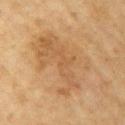This lesion was catalogued during total-body skin photography and was not selected for biopsy. The lesion is located on the right upper arm. This is a cross-polarized tile. The lesion's longest dimension is about 8 mm. The subject is a female aged approximately 55. An algorithmic analysis of the crop reported an average lesion color of about L≈51 a*≈17 b*≈35 (CIELAB), a lesion–skin lightness drop of about 7, and a normalized border contrast of about 5.5. It also reported a nevus-likeness score of about 20/100 and a detector confidence of about 100 out of 100 that the crop contains a lesion. This image is a 15 mm lesion crop taken from a total-body photograph.